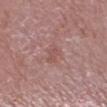| field | value |
|---|---|
| follow-up | imaged on a skin check; not biopsied |
| location | the left lower leg |
| illumination | white-light |
| patient | male, approximately 85 years of age or older |
| image source | ~15 mm tile from a whole-body skin photo |
| lesion size | ~3 mm (longest diameter) |